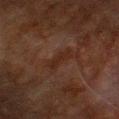patient = male, about 80 years old; lesion diameter = ~3 mm (longest diameter); site = the chest; image source = 15 mm crop, total-body photography.